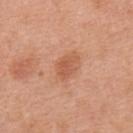Q: Is there a histopathology result?
A: catalogued during a skin exam; not biopsied
Q: How large is the lesion?
A: about 3 mm
Q: What is the imaging modality?
A: 15 mm crop, total-body photography
Q: Who is the patient?
A: male, aged 68 to 72
Q: Where on the body is the lesion?
A: the back
Q: Automated lesion metrics?
A: an area of roughly 5 mm², an outline eccentricity of about 0.75 (0 = round, 1 = elongated), and two-axis asymmetry of about 0.25; a nevus-likeness score of about 75/100 and lesion-presence confidence of about 100/100